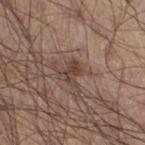{"biopsy_status": "not biopsied; imaged during a skin examination", "site": "leg", "lighting": "white-light", "image": {"source": "total-body photography crop", "field_of_view_mm": 15}, "lesion_size": {"long_diameter_mm_approx": 3.0}, "automated_metrics": {"area_mm2_approx": 4.0, "eccentricity": 0.7, "shape_asymmetry": 0.45, "cielab_L": 41, "cielab_a": 17, "cielab_b": 23, "vs_skin_darker_L": 9.0, "vs_skin_contrast_norm": 7.5, "border_irregularity_0_10": 5.5, "color_variation_0_10": 1.0, "peripheral_color_asymmetry": 0.5, "nevus_likeness_0_100": 5}, "patient": {"sex": "male", "age_approx": 55}}